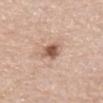notes: no biopsy performed (imaged during a skin exam)
illumination: white-light
automated lesion analysis: a footprint of about 5 mm² and a shape-asymmetry score of about 0.3 (0 = symmetric); a classifier nevus-likeness of about 85/100
acquisition: ~15 mm tile from a whole-body skin photo
size: ~3 mm (longest diameter)
site: the mid back
patient: male, aged 73 to 77